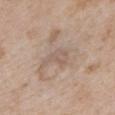Case summary:
* patient — female, about 30 years old
* illumination — white-light
* body site — the mid back
* lesion diameter — ≈3.5 mm
* image — 15 mm crop, total-body photography